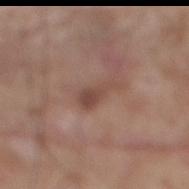Findings:
– follow-up · no biopsy performed (imaged during a skin exam)
– image · ~15 mm tile from a whole-body skin photo
– anatomic site · the mid back
– subject · male, about 60 years old